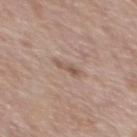| feature | finding |
|---|---|
| notes | catalogued during a skin exam; not biopsied |
| site | the chest |
| image | ~15 mm crop, total-body skin-cancer survey |
| patient | male, approximately 70 years of age |